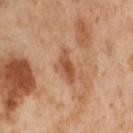notes: total-body-photography surveillance lesion; no biopsy | anatomic site: the right thigh | image source: total-body-photography crop, ~15 mm field of view | subject: female, roughly 55 years of age | TBP lesion metrics: about 10 CIELAB-L* units darker than the surrounding skin and a normalized border contrast of about 7.5; radial color variation of about 1.